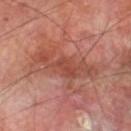This lesion was catalogued during total-body skin photography and was not selected for biopsy.